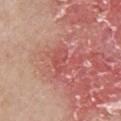A region of skin cropped from a whole-body photographic capture, roughly 15 mm wide. From the chest. A male patient, aged 68 to 72. The total-body-photography lesion software estimated an average lesion color of about L≈52 a*≈32 b*≈27 (CIELAB) and a lesion-to-skin contrast of about 4.5 (normalized; higher = more distinct). It also reported border irregularity of about 4.5 on a 0–10 scale, a color-variation rating of about 2/10, and peripheral color asymmetry of about 0.5. The recorded lesion diameter is about 3 mm. Imaged with white-light lighting. On excision, pathology confirmed an actinic keratosis — a lesion of indeterminate malignant potential.